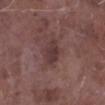Imaged during a routine full-body skin examination; the lesion was not biopsied and no histopathology is available.
On the right lower leg.
Captured under white-light illumination.
The recorded lesion diameter is about 3.5 mm.
A 15 mm crop from a total-body photograph taken for skin-cancer surveillance.
The patient is a male about 75 years old.